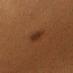Part of a total-body skin-imaging series; this lesion was reviewed on a skin check and was not flagged for biopsy. A roughly 15 mm field-of-view crop from a total-body skin photograph. From the arm. The lesion's longest dimension is about 2.5 mm. A female patient, in their mid- to late 50s. The tile uses cross-polarized illumination. The total-body-photography lesion software estimated a footprint of about 5 mm² and a shape eccentricity near 0.6. And it measured a classifier nevus-likeness of about 95/100.